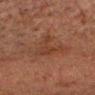Imaged during a routine full-body skin examination; the lesion was not biopsied and no histopathology is available. On the head or neck. This is a cross-polarized tile. The total-body-photography lesion software estimated an area of roughly 7.5 mm², an outline eccentricity of about 0.6 (0 = round, 1 = elongated), and a shape-asymmetry score of about 0.7 (0 = symmetric). The analysis additionally found a lesion color around L≈29 a*≈18 b*≈24 in CIELAB and about 5 CIELAB-L* units darker than the surrounding skin. The lesion's longest dimension is about 4 mm. The patient is a male in their 60s. A lesion tile, about 15 mm wide, cut from a 3D total-body photograph.